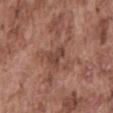{
  "site": "mid back",
  "patient": {
    "sex": "male",
    "age_approx": 75
  },
  "automated_metrics": {
    "area_mm2_approx": 4.0,
    "eccentricity": 0.65,
    "shape_asymmetry": 0.4,
    "vs_skin_darker_L": 8.0,
    "vs_skin_contrast_norm": 7.0
  },
  "image": {
    "source": "total-body photography crop",
    "field_of_view_mm": 15
  },
  "lighting": "white-light"
}